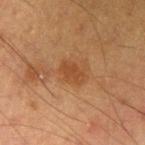No biopsy was performed on this lesion — it was imaged during a full skin examination and was not determined to be concerning. Imaged with cross-polarized lighting. A male subject aged around 55. The recorded lesion diameter is about 3 mm. This image is a 15 mm lesion crop taken from a total-body photograph. Automated image analysis of the tile measured an outline eccentricity of about 0.75 (0 = round, 1 = elongated) and a shape-asymmetry score of about 0.25 (0 = symmetric). The software also gave an automated nevus-likeness rating near 45 out of 100. Located on the right upper arm.